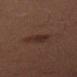Assessment: Recorded during total-body skin imaging; not selected for excision or biopsy. Context: A male subject aged around 45. Located on the left thigh. The lesion's longest dimension is about 3 mm. Automated image analysis of the tile measured a footprint of about 6 mm², an outline eccentricity of about 0.7 (0 = round, 1 = elongated), and a shape-asymmetry score of about 0.15 (0 = symmetric). And it measured an average lesion color of about L≈22 a*≈14 b*≈18 (CIELAB), roughly 5 lightness units darker than nearby skin, and a normalized border contrast of about 6.5. And it measured a border-irregularity rating of about 1.5/10, internal color variation of about 2.5 on a 0–10 scale, and peripheral color asymmetry of about 1. A close-up tile cropped from a whole-body skin photograph, about 15 mm across.